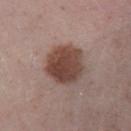Q: Is there a histopathology result?
A: catalogued during a skin exam; not biopsied
Q: What are the patient's age and sex?
A: male, aged 48–52
Q: Illumination type?
A: white-light
Q: How large is the lesion?
A: ~5 mm (longest diameter)
Q: Where on the body is the lesion?
A: the leg
Q: What kind of image is this?
A: total-body-photography crop, ~15 mm field of view
Q: Automated lesion metrics?
A: a mean CIELAB color near L≈44 a*≈19 b*≈23 and a normalized border contrast of about 10.5; an automated nevus-likeness rating near 100 out of 100 and a detector confidence of about 100 out of 100 that the crop contains a lesion